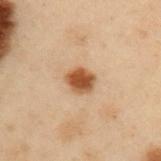Assessment:
Part of a total-body skin-imaging series; this lesion was reviewed on a skin check and was not flagged for biopsy.
Context:
The lesion-visualizer software estimated roughly 14 lightness units darker than nearby skin and a normalized border contrast of about 11. And it measured border irregularity of about 2 on a 0–10 scale and a peripheral color-asymmetry measure near 1. It also reported an automated nevus-likeness rating near 100 out of 100 and a detector confidence of about 100 out of 100 that the crop contains a lesion. This image is a 15 mm lesion crop taken from a total-body photograph. A male subject, aged 53 to 57. Imaged with cross-polarized lighting. From the arm. About 3 mm across.